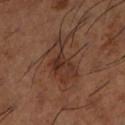Impression: No biopsy was performed on this lesion — it was imaged during a full skin examination and was not determined to be concerning. Acquisition and patient details: Cropped from a total-body skin-imaging series; the visible field is about 15 mm. The lesion is located on the leg. Imaged with cross-polarized lighting. A male subject aged 63–67.